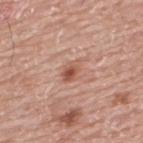Clinical impression: This lesion was catalogued during total-body skin photography and was not selected for biopsy. Clinical summary: This image is a 15 mm lesion crop taken from a total-body photograph. The tile uses white-light illumination. The recorded lesion diameter is about 2.5 mm. A male patient, aged 73–77. From the upper back. The total-body-photography lesion software estimated a lesion area of about 4 mm² and an outline eccentricity of about 0.75 (0 = round, 1 = elongated). The analysis additionally found a lesion color around L≈54 a*≈24 b*≈29 in CIELAB and a lesion-to-skin contrast of about 7.5 (normalized; higher = more distinct). The software also gave peripheral color asymmetry of about 1.5.